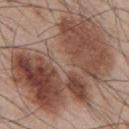No biopsy was performed on this lesion — it was imaged during a full skin examination and was not determined to be concerning. Captured under white-light illumination. The recorded lesion diameter is about 13 mm. From the upper back. The patient is a male in their mid-50s. Cropped from a total-body skin-imaging series; the visible field is about 15 mm.